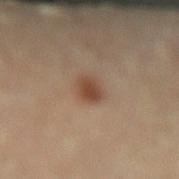follow-up: no biopsy performed (imaged during a skin exam) | tile lighting: cross-polarized | size: about 3 mm | imaging modality: ~15 mm tile from a whole-body skin photo | patient: female, about 60 years old | location: the left lower leg.